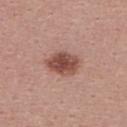{
  "lighting": "white-light",
  "lesion_size": {
    "long_diameter_mm_approx": 4.0
  },
  "patient": {
    "sex": "female",
    "age_approx": 25
  },
  "site": "upper back",
  "image": {
    "source": "total-body photography crop",
    "field_of_view_mm": 15
  },
  "automated_metrics": {
    "vs_skin_darker_L": 14.0,
    "vs_skin_contrast_norm": 10.0,
    "nevus_likeness_0_100": 95
  }
}